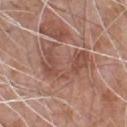Q: How was this image acquired?
A: ~15 mm tile from a whole-body skin photo
Q: Lesion size?
A: about 5.5 mm
Q: What lighting was used for the tile?
A: white-light illumination
Q: Lesion location?
A: the chest
Q: Patient demographics?
A: male, aged around 65